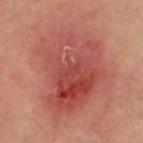<case>
<biopsy_status>not biopsied; imaged during a skin examination</biopsy_status>
<automated_metrics>
  <lesion_detection_confidence_0_100>100</lesion_detection_confidence_0_100>
</automated_metrics>
<image>
  <source>total-body photography crop</source>
  <field_of_view_mm>15</field_of_view_mm>
</image>
<site>upper back</site>
<lesion_size>
  <long_diameter_mm_approx>9.5</long_diameter_mm_approx>
</lesion_size>
<patient>
  <sex>female</sex>
  <age_approx>55</age_approx>
</patient>
</case>